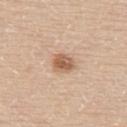Context:
The tile uses white-light illumination. Automated tile analysis of the lesion measured a symmetry-axis asymmetry near 0.2. The analysis additionally found about 12 CIELAB-L* units darker than the surrounding skin. And it measured a border-irregularity rating of about 1.5/10, internal color variation of about 3.5 on a 0–10 scale, and a peripheral color-asymmetry measure near 1. The software also gave a nevus-likeness score of about 95/100 and a detector confidence of about 100 out of 100 that the crop contains a lesion. A male patient in their 60s. A region of skin cropped from a whole-body photographic capture, roughly 15 mm wide. Longest diameter approximately 2.5 mm. The lesion is located on the upper back.